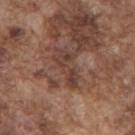The lesion was tiled from a total-body skin photograph and was not biopsied. The recorded lesion diameter is about 4.5 mm. The tile uses white-light illumination. A lesion tile, about 15 mm wide, cut from a 3D total-body photograph. A male patient, aged approximately 75. The lesion is located on the back.